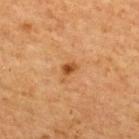Imaged during a routine full-body skin examination; the lesion was not biopsied and no histopathology is available. From the back. Captured under cross-polarized illumination. Automated image analysis of the tile measured a border-irregularity rating of about 4.5/10, a within-lesion color-variation index near 2.5/10, and a peripheral color-asymmetry measure near 0.5. And it measured a nevus-likeness score of about 70/100 and a detector confidence of about 100 out of 100 that the crop contains a lesion. This image is a 15 mm lesion crop taken from a total-body photograph. Measured at roughly 2.5 mm in maximum diameter. A male patient, approximately 65 years of age.